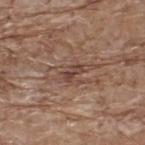Recorded during total-body skin imaging; not selected for excision or biopsy. Automated tile analysis of the lesion measured a within-lesion color-variation index near 1.5/10 and peripheral color asymmetry of about 0.5. And it measured an automated nevus-likeness rating near 0 out of 100 and lesion-presence confidence of about 90/100. On the upper back. The subject is a male approximately 70 years of age. Cropped from a total-body skin-imaging series; the visible field is about 15 mm. This is a white-light tile.